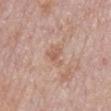Notes:
- biopsy status · imaged on a skin check; not biopsied
- acquisition · ~15 mm tile from a whole-body skin photo
- TBP lesion metrics · an average lesion color of about L≈59 a*≈20 b*≈29 (CIELAB), about 8 CIELAB-L* units darker than the surrounding skin, and a lesion-to-skin contrast of about 5.5 (normalized; higher = more distinct); radial color variation of about 0.5; a nevus-likeness score of about 0/100 and a lesion-detection confidence of about 100/100
- illumination · white-light illumination
- size · ≈3 mm
- patient · male, about 75 years old
- location · the mid back